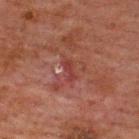follow-up: imaged on a skin check; not biopsied
anatomic site: the upper back
patient: male, about 60 years old
lighting: cross-polarized
diameter: ~2.5 mm (longest diameter)
image: 15 mm crop, total-body photography
automated metrics: a border-irregularity rating of about 4.5/10, a within-lesion color-variation index near 0/10, and radial color variation of about 0